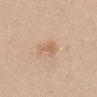| field | value |
|---|---|
| follow-up | catalogued during a skin exam; not biopsied |
| illumination | white-light |
| lesion diameter | ≈2.5 mm |
| anatomic site | the back |
| image | total-body-photography crop, ~15 mm field of view |
| patient | female, aged around 30 |